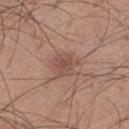* follow-up · imaged on a skin check; not biopsied
* body site · the left lower leg
* lesion size · ≈3.5 mm
* acquisition · 15 mm crop, total-body photography
* subject · male, approximately 30 years of age
* image-analysis metrics · a lesion color around L≈50 a*≈21 b*≈24 in CIELAB and a lesion–skin lightness drop of about 8; a border-irregularity index near 4.5/10, a color-variation rating of about 2/10, and radial color variation of about 0.5
* illumination · white-light